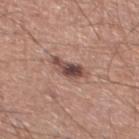Clinical impression:
Captured during whole-body skin photography for melanoma surveillance; the lesion was not biopsied.
Background:
A 15 mm close-up extracted from a 3D total-body photography capture. A male subject, aged 38 to 42. The lesion is on the leg.